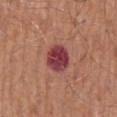No biopsy was performed on this lesion — it was imaged during a full skin examination and was not determined to be concerning.